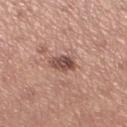Assessment: Part of a total-body skin-imaging series; this lesion was reviewed on a skin check and was not flagged for biopsy. Image and clinical context: Located on the left lower leg. A region of skin cropped from a whole-body photographic capture, roughly 15 mm wide. Imaged with white-light lighting. About 3 mm across. The subject is a female approximately 65 years of age. The lesion-visualizer software estimated a footprint of about 5 mm² and a shape eccentricity near 0.75.